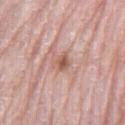biopsy status: imaged on a skin check; not biopsied